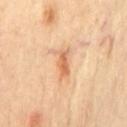biopsy status: catalogued during a skin exam; not biopsied
image: ~15 mm tile from a whole-body skin photo
lesion size: ≈3.5 mm
site: the front of the torso